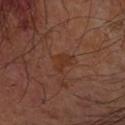Notes:
– follow-up · no biopsy performed (imaged during a skin exam)
– subject · male, roughly 65 years of age
– automated lesion analysis · a footprint of about 3.5 mm² and a shape-asymmetry score of about 0.3 (0 = symmetric); a mean CIELAB color near L≈31 a*≈21 b*≈27 and a lesion-to-skin contrast of about 6 (normalized; higher = more distinct)
– lesion diameter · ~2.5 mm (longest diameter)
– site · the arm
– lighting · cross-polarized illumination
– acquisition · 15 mm crop, total-body photography